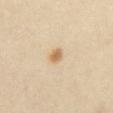Q: Is there a histopathology result?
A: catalogued during a skin exam; not biopsied
Q: What is the imaging modality?
A: ~15 mm tile from a whole-body skin photo
Q: Illumination type?
A: cross-polarized
Q: Lesion location?
A: the abdomen
Q: Who is the patient?
A: female, about 50 years old
Q: Automated lesion metrics?
A: a lesion–skin lightness drop of about 11 and a normalized lesion–skin contrast near 7.5
Q: How large is the lesion?
A: ~2 mm (longest diameter)